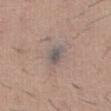| key | value |
|---|---|
| notes | catalogued during a skin exam; not biopsied |
| patient | male, in their 60s |
| acquisition | ~15 mm tile from a whole-body skin photo |
| site | the abdomen |